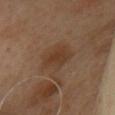Assessment:
The lesion was tiled from a total-body skin photograph and was not biopsied.
Acquisition and patient details:
A female patient approximately 60 years of age. The lesion's longest dimension is about 3.5 mm. From the head or neck. A 15 mm crop from a total-body photograph taken for skin-cancer surveillance.